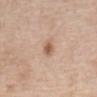An algorithmic analysis of the crop reported a border-irregularity index near 2/10 and a color-variation rating of about 2.5/10. The software also gave a nevus-likeness score of about 80/100 and lesion-presence confidence of about 100/100. The patient is a female aged approximately 65. The lesion is located on the abdomen. A roughly 15 mm field-of-view crop from a total-body skin photograph. The recorded lesion diameter is about 2.5 mm.